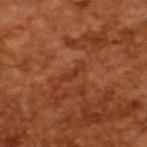Findings:
* workup · catalogued during a skin exam; not biopsied
* subject · male, aged 63–67
* image · total-body-photography crop, ~15 mm field of view